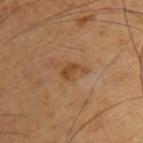Impression: The lesion was tiled from a total-body skin photograph and was not biopsied. Clinical summary: Automated image analysis of the tile measured two-axis asymmetry of about 0.45. And it measured a mean CIELAB color near L≈43 a*≈20 b*≈35 and a normalized border contrast of about 7.5. The software also gave a border-irregularity rating of about 5.5/10, a within-lesion color-variation index near 1/10, and a peripheral color-asymmetry measure near 0.5. It also reported a classifier nevus-likeness of about 5/100 and a lesion-detection confidence of about 100/100. A male patient, aged 58–62. The lesion's longest dimension is about 3 mm. A close-up tile cropped from a whole-body skin photograph, about 15 mm across. On the upper back.